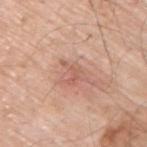Clinical impression:
No biopsy was performed on this lesion — it was imaged during a full skin examination and was not determined to be concerning.
Acquisition and patient details:
A 15 mm crop from a total-body photograph taken for skin-cancer surveillance. The subject is a male approximately 70 years of age. Located on the upper back.